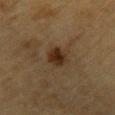{"biopsy_status": "not biopsied; imaged during a skin examination", "image": {"source": "total-body photography crop", "field_of_view_mm": 15}, "lighting": "cross-polarized", "lesion_size": {"long_diameter_mm_approx": 3.5}, "patient": {"sex": "male", "age_approx": "85+"}, "site": "arm"}